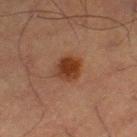Case summary:
- follow-up: catalogued during a skin exam; not biopsied
- imaging modality: total-body-photography crop, ~15 mm field of view
- subject: male, in their mid-60s
- lesion size: ≈3 mm
- anatomic site: the right thigh